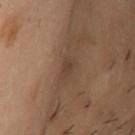  biopsy_status: not biopsied; imaged during a skin examination
  patient:
    sex: female
    age_approx: 55
  lesion_size:
    long_diameter_mm_approx: 2.5
  lighting: cross-polarized
  site: arm
  image:
    source: total-body photography crop
    field_of_view_mm: 15
  automated_metrics:
    area_mm2_approx: 3.5
    eccentricity: 0.8
    shape_asymmetry: 0.35
    border_irregularity_0_10: 3.0
    color_variation_0_10: 1.5
    peripheral_color_asymmetry: 0.5